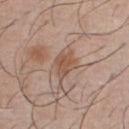Recorded during total-body skin imaging; not selected for excision or biopsy. Cropped from a whole-body photographic skin survey; the tile spans about 15 mm. The lesion is on the chest. A male subject, aged approximately 45. About 3 mm across. This is a white-light tile.